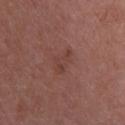Notes:
– follow-up: total-body-photography surveillance lesion; no biopsy
– image: ~15 mm crop, total-body skin-cancer survey
– lighting: white-light illumination
– subject: female, aged around 70
– anatomic site: the head or neck
– automated lesion analysis: an area of roughly 4.5 mm², an outline eccentricity of about 0.85 (0 = round, 1 = elongated), and a symmetry-axis asymmetry near 0.45
– lesion size: ≈3.5 mm